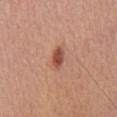Imaged during a routine full-body skin examination; the lesion was not biopsied and no histopathology is available.
The lesion is located on the chest.
The patient is a male roughly 65 years of age.
Automated tile analysis of the lesion measured a lesion area of about 4 mm². The software also gave an average lesion color of about L≈51 a*≈26 b*≈30 (CIELAB) and about 13 CIELAB-L* units darker than the surrounding skin. The analysis additionally found a border-irregularity index near 2/10, internal color variation of about 3 on a 0–10 scale, and peripheral color asymmetry of about 1. The analysis additionally found a nevus-likeness score of about 95/100 and lesion-presence confidence of about 100/100.
The recorded lesion diameter is about 3 mm.
A 15 mm close-up tile from a total-body photography series done for melanoma screening.